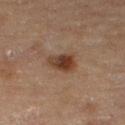follow-up: imaged on a skin check; not biopsied | imaging modality: ~15 mm tile from a whole-body skin photo | subject: female, aged 53 to 57 | diameter: ≈4 mm | site: the leg.